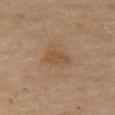The lesion was tiled from a total-body skin photograph and was not biopsied. About 3 mm across. Located on the left thigh. Cropped from a total-body skin-imaging series; the visible field is about 15 mm. Automated image analysis of the tile measured a border-irregularity rating of about 4/10, a color-variation rating of about 1/10, and peripheral color asymmetry of about 0.5. The software also gave a nevus-likeness score of about 5/100 and a lesion-detection confidence of about 100/100. This is a cross-polarized tile. The patient is a female aged 58–62.